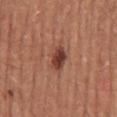Notes:
- notes: catalogued during a skin exam; not biopsied
- lesion size: about 3 mm
- body site: the front of the torso
- acquisition: total-body-photography crop, ~15 mm field of view
- tile lighting: white-light illumination
- subject: male, aged around 45